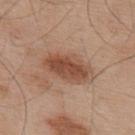Assessment:
Part of a total-body skin-imaging series; this lesion was reviewed on a skin check and was not flagged for biopsy.
Image and clinical context:
Longest diameter approximately 5.5 mm. Located on the upper back. Cropped from a whole-body photographic skin survey; the tile spans about 15 mm. A male patient aged 53–57. Imaged with white-light lighting.